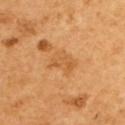<tbp_lesion>
  <biopsy_status>not biopsied; imaged during a skin examination</biopsy_status>
  <automated_metrics>
    <cielab_L>59</cielab_L>
    <cielab_a>24</cielab_a>
    <cielab_b>45</cielab_b>
    <vs_skin_contrast_norm>5.0</vs_skin_contrast_norm>
  </automated_metrics>
  <image>
    <source>total-body photography crop</source>
    <field_of_view_mm>15</field_of_view_mm>
  </image>
  <site>upper back</site>
  <lesion_size>
    <long_diameter_mm_approx>3.5</long_diameter_mm_approx>
  </lesion_size>
  <patient>
    <sex>female</sex>
    <age_approx>55</age_approx>
  </patient>
  <lighting>cross-polarized</lighting>
</tbp_lesion>